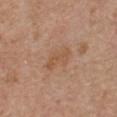biopsy status — catalogued during a skin exam; not biopsied | diameter — ≈4 mm | site — the chest | acquisition — total-body-photography crop, ~15 mm field of view | automated lesion analysis — a within-lesion color-variation index near 2/10 and a peripheral color-asymmetry measure near 0.5; an automated nevus-likeness rating near 0 out of 100 and a detector confidence of about 100 out of 100 that the crop contains a lesion | subject — male, aged approximately 80.